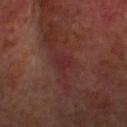Background:
The lesion is on the head or neck. About 3.5 mm across. Captured under cross-polarized illumination. A 15 mm close-up extracted from a 3D total-body photography capture. Automated image analysis of the tile measured an area of roughly 4.5 mm², a shape eccentricity near 0.75, and a shape-asymmetry score of about 0.4 (0 = symmetric). The software also gave about 5 CIELAB-L* units darker than the surrounding skin. The software also gave a border-irregularity rating of about 4.5/10, a within-lesion color-variation index near 1/10, and peripheral color asymmetry of about 0.5. A male subject about 65 years old.